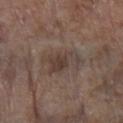The lesion is on the left forearm. Imaged with white-light lighting. Automated tile analysis of the lesion measured a border-irregularity rating of about 3.5/10, a color-variation rating of about 4.5/10, and peripheral color asymmetry of about 1.5. The software also gave an automated nevus-likeness rating near 35 out of 100 and a detector confidence of about 95 out of 100 that the crop contains a lesion. A 15 mm close-up tile from a total-body photography series done for melanoma screening. A female patient aged around 85 or older. The lesion's longest dimension is about 4.5 mm.